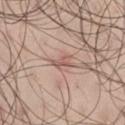Captured during whole-body skin photography for melanoma surveillance; the lesion was not biopsied.
This is a white-light tile.
Located on the leg.
A close-up tile cropped from a whole-body skin photograph, about 15 mm across.
The subject is a male about 45 years old.
Longest diameter approximately 2.5 mm.